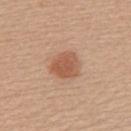The subject is a female aged 38–42. The lesion is located on the upper back. The recorded lesion diameter is about 3.5 mm. Imaged with white-light lighting. Cropped from a total-body skin-imaging series; the visible field is about 15 mm.